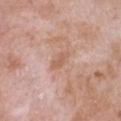Notes:
• notes — imaged on a skin check; not biopsied
• acquisition — ~15 mm crop, total-body skin-cancer survey
• image-analysis metrics — a lesion area of about 4 mm², an eccentricity of roughly 0.85, and a shape-asymmetry score of about 0.2 (0 = symmetric); a border-irregularity index near 2/10, internal color variation of about 2 on a 0–10 scale, and peripheral color asymmetry of about 0.5
• illumination — white-light illumination
• patient — male, roughly 50 years of age
• size — about 3 mm
• body site — the chest